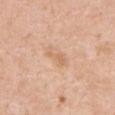Case summary:
* notes: no biopsy performed (imaged during a skin exam)
* lighting: white-light
* patient: female, aged around 40
* location: the chest
* lesion size: ~3 mm (longest diameter)
* image source: ~15 mm tile from a whole-body skin photo